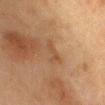No biopsy was performed on this lesion — it was imaged during a full skin examination and was not determined to be concerning. A 15 mm close-up extracted from a 3D total-body photography capture. A female patient, approximately 50 years of age. Measured at roughly 3 mm in maximum diameter. From the chest.